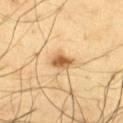The lesion was photographed on a routine skin check and not biopsied; there is no pathology result.
The total-body-photography lesion software estimated an area of roughly 4.5 mm², a shape eccentricity near 0.75, and a shape-asymmetry score of about 0.25 (0 = symmetric). It also reported an average lesion color of about L≈63 a*≈21 b*≈43 (CIELAB), about 15 CIELAB-L* units darker than the surrounding skin, and a normalized lesion–skin contrast near 9.5. The analysis additionally found a nevus-likeness score of about 95/100 and a detector confidence of about 100 out of 100 that the crop contains a lesion.
A male subject aged approximately 65.
The lesion is on the right thigh.
Approximately 3 mm at its widest.
A 15 mm close-up extracted from a 3D total-body photography capture.
This is a cross-polarized tile.